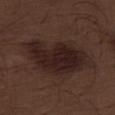Captured during whole-body skin photography for melanoma surveillance; the lesion was not biopsied. The subject is a male aged 68–72. A 15 mm close-up extracted from a 3D total-body photography capture. The lesion is on the right thigh.